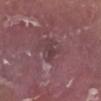biopsy status: catalogued during a skin exam; not biopsied | patient: male, in their mid-60s | tile lighting: white-light illumination | body site: the left lower leg | lesion size: about 2.5 mm | imaging modality: ~15 mm crop, total-body skin-cancer survey.